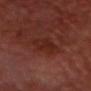{"biopsy_status": "not biopsied; imaged during a skin examination", "patient": {"sex": "male", "age_approx": 50}, "image": {"source": "total-body photography crop", "field_of_view_mm": 15}, "lighting": "cross-polarized", "site": "head or neck", "lesion_size": {"long_diameter_mm_approx": 3.5}}